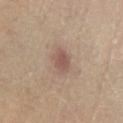{
  "biopsy_status": "not biopsied; imaged during a skin examination",
  "automated_metrics": {
    "nevus_likeness_0_100": 35,
    "lesion_detection_confidence_0_100": 100
  },
  "site": "left lower leg",
  "patient": {
    "sex": "male",
    "age_approx": 60
  },
  "lighting": "cross-polarized",
  "image": {
    "source": "total-body photography crop",
    "field_of_view_mm": 15
  },
  "lesion_size": {
    "long_diameter_mm_approx": 3.0
  }
}